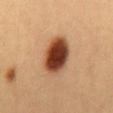This lesion was catalogued during total-body skin photography and was not selected for biopsy.
Imaged with cross-polarized lighting.
A roughly 15 mm field-of-view crop from a total-body skin photograph.
The recorded lesion diameter is about 5.5 mm.
From the abdomen.
The patient is a female aged 23–27.
The lesion-visualizer software estimated a shape eccentricity near 0.75 and two-axis asymmetry of about 0.1. It also reported a within-lesion color-variation index near 8/10 and a peripheral color-asymmetry measure near 2.